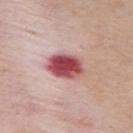A male subject roughly 65 years of age.
About 4.5 mm across.
The lesion is located on the back.
Automated image analysis of the tile measured roughly 20 lightness units darker than nearby skin and a normalized lesion–skin contrast near 12.5. The analysis additionally found border irregularity of about 1.5 on a 0–10 scale, a within-lesion color-variation index near 6.5/10, and a peripheral color-asymmetry measure near 2. The analysis additionally found a lesion-detection confidence of about 100/100.
A lesion tile, about 15 mm wide, cut from a 3D total-body photograph.
Imaged with white-light lighting.